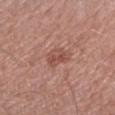Part of a total-body skin-imaging series; this lesion was reviewed on a skin check and was not flagged for biopsy. On the right forearm. A female subject aged 58–62. Cropped from a total-body skin-imaging series; the visible field is about 15 mm. The recorded lesion diameter is about 3 mm.